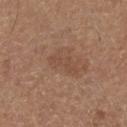– notes: no biopsy performed (imaged during a skin exam)
– automated lesion analysis: a lesion color around L≈47 a*≈19 b*≈29 in CIELAB, roughly 6 lightness units darker than nearby skin, and a lesion-to-skin contrast of about 4.5 (normalized; higher = more distinct); an automated nevus-likeness rating near 0 out of 100 and lesion-presence confidence of about 100/100
– subject: male, aged around 75
– tile lighting: white-light
– imaging modality: total-body-photography crop, ~15 mm field of view
– diameter: ≈3.5 mm
– location: the right lower leg